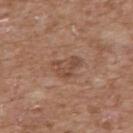This lesion was catalogued during total-body skin photography and was not selected for biopsy. Located on the chest. A male subject about 60 years old. An algorithmic analysis of the crop reported a mean CIELAB color near L≈48 a*≈20 b*≈28, roughly 8 lightness units darker than nearby skin, and a normalized border contrast of about 6. The analysis additionally found internal color variation of about 3 on a 0–10 scale. The software also gave a detector confidence of about 100 out of 100 that the crop contains a lesion. This is a white-light tile. Cropped from a whole-body photographic skin survey; the tile spans about 15 mm. The recorded lesion diameter is about 3.5 mm.